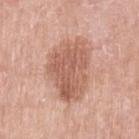Q: Was a biopsy performed?
A: no biopsy performed (imaged during a skin exam)
Q: What is the imaging modality?
A: 15 mm crop, total-body photography
Q: Where on the body is the lesion?
A: the right upper arm
Q: What did automated image analysis measure?
A: a mean CIELAB color near L≈59 a*≈23 b*≈29, about 11 CIELAB-L* units darker than the surrounding skin, and a normalized lesion–skin contrast near 7; a within-lesion color-variation index near 4.5/10 and a peripheral color-asymmetry measure near 2; an automated nevus-likeness rating near 0 out of 100 and lesion-presence confidence of about 100/100
Q: What lighting was used for the tile?
A: white-light
Q: Who is the patient?
A: female, aged 63–67
Q: How large is the lesion?
A: ~7 mm (longest diameter)